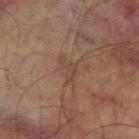Impression:
The lesion was tiled from a total-body skin photograph and was not biopsied.
Clinical summary:
The lesion is located on the left forearm. This is a cross-polarized tile. A lesion tile, about 15 mm wide, cut from a 3D total-body photograph. The patient is a male roughly 75 years of age. The recorded lesion diameter is about 3 mm. The lesion-visualizer software estimated an automated nevus-likeness rating near 0 out of 100 and a lesion-detection confidence of about 95/100.